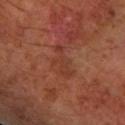No biopsy was performed on this lesion — it was imaged during a full skin examination and was not determined to be concerning. Automated tile analysis of the lesion measured a footprint of about 5 mm² and a shape eccentricity near 0.9. It also reported a lesion color around L≈36 a*≈25 b*≈29 in CIELAB. The software also gave a border-irregularity index near 7.5/10 and a peripheral color-asymmetry measure near 0. The software also gave a classifier nevus-likeness of about 0/100 and lesion-presence confidence of about 100/100. A 15 mm close-up tile from a total-body photography series done for melanoma screening. The lesion is located on the left forearm. About 4 mm across. This is a cross-polarized tile. A male subject, roughly 60 years of age.